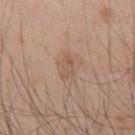Recorded during total-body skin imaging; not selected for excision or biopsy. This image is a 15 mm lesion crop taken from a total-body photograph. From the upper back. Automated tile analysis of the lesion measured an outline eccentricity of about 0.9 (0 = round, 1 = elongated) and a symmetry-axis asymmetry near 0.4. It also reported a border-irregularity index near 4/10, a within-lesion color-variation index near 2/10, and a peripheral color-asymmetry measure near 0. The analysis additionally found an automated nevus-likeness rating near 5 out of 100. The patient is a male about 45 years old.